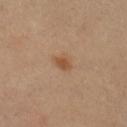Imaged during a routine full-body skin examination; the lesion was not biopsied and no histopathology is available.
The recorded lesion diameter is about 2.5 mm.
This is a cross-polarized tile.
The lesion is on the right lower leg.
A 15 mm close-up tile from a total-body photography series done for melanoma screening.
A female patient about 50 years old.
Automated image analysis of the tile measured a footprint of about 3 mm², a shape eccentricity near 0.75, and two-axis asymmetry of about 0.25. The analysis additionally found a lesion color around L≈49 a*≈19 b*≈33 in CIELAB and a lesion–skin lightness drop of about 8.